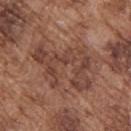Assessment: Part of a total-body skin-imaging series; this lesion was reviewed on a skin check and was not flagged for biopsy. Background: Captured under white-light illumination. Located on the upper back. A 15 mm crop from a total-body photograph taken for skin-cancer surveillance. The patient is a male in their mid-70s. Measured at roughly 7.5 mm in maximum diameter.